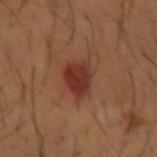Captured during whole-body skin photography for melanoma surveillance; the lesion was not biopsied. On the left forearm. The subject is a male aged 63–67. A 15 mm close-up extracted from a 3D total-body photography capture. The lesion's longest dimension is about 4 mm.